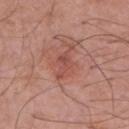Part of a total-body skin-imaging series; this lesion was reviewed on a skin check and was not flagged for biopsy. A lesion tile, about 15 mm wide, cut from a 3D total-body photograph. The lesion is on the left upper arm. An algorithmic analysis of the crop reported an area of roughly 4 mm² and an eccentricity of roughly 0.9. And it measured a lesion color around L≈50 a*≈26 b*≈26 in CIELAB, a lesion–skin lightness drop of about 8, and a normalized border contrast of about 5.5. The recorded lesion diameter is about 3.5 mm. A male patient about 70 years old.